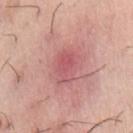| field | value |
|---|---|
| notes | imaged on a skin check; not biopsied |
| patient | male, aged 73–77 |
| site | the chest |
| image source | ~15 mm tile from a whole-body skin photo |